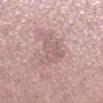Findings:
– subject · female, aged 38 to 42
– image source · ~15 mm crop, total-body skin-cancer survey
– lighting · white-light illumination
– size · about 5.5 mm
– location · the right lower leg